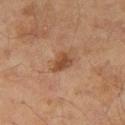Part of a total-body skin-imaging series; this lesion was reviewed on a skin check and was not flagged for biopsy.
The tile uses cross-polarized illumination.
A roughly 15 mm field-of-view crop from a total-body skin photograph.
A male patient, aged 58 to 62.
From the right thigh.
About 3 mm across.